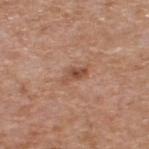biopsy status: catalogued during a skin exam; not biopsied | anatomic site: the back | patient: male, roughly 60 years of age | tile lighting: white-light | image source: 15 mm crop, total-body photography.